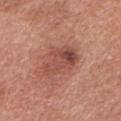Q: Is there a histopathology result?
A: total-body-photography surveillance lesion; no biopsy
Q: Who is the patient?
A: female, in their mid-70s
Q: What did automated image analysis measure?
A: a lesion area of about 13 mm² and an outline eccentricity of about 0.75 (0 = round, 1 = elongated); an average lesion color of about L≈49 a*≈25 b*≈28 (CIELAB), a lesion–skin lightness drop of about 10, and a normalized border contrast of about 7; a border-irregularity index near 3.5/10, a within-lesion color-variation index near 6.5/10, and radial color variation of about 2.5; a nevus-likeness score of about 20/100 and a detector confidence of about 100 out of 100 that the crop contains a lesion
Q: What is the lesion's diameter?
A: ~5 mm (longest diameter)
Q: What is the imaging modality?
A: 15 mm crop, total-body photography
Q: What is the anatomic site?
A: the head or neck